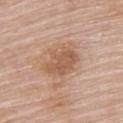biopsy status: catalogued during a skin exam; not biopsied | location: the upper back | diameter: ~5 mm (longest diameter) | tile lighting: white-light | automated metrics: an area of roughly 13 mm², an outline eccentricity of about 0.7 (0 = round, 1 = elongated), and a symmetry-axis asymmetry near 0.25; an automated nevus-likeness rating near 5 out of 100 and a lesion-detection confidence of about 100/100 | patient: female, in their mid-60s | image: 15 mm crop, total-body photography.